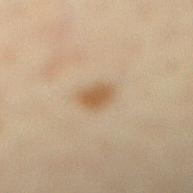<case>
  <biopsy_status>not biopsied; imaged during a skin examination</biopsy_status>
  <automated_metrics>
    <border_irregularity_0_10>2.0</border_irregularity_0_10>
    <color_variation_0_10>2.0</color_variation_0_10>
    <peripheral_color_asymmetry>0.5</peripheral_color_asymmetry>
  </automated_metrics>
  <patient>
    <sex>female</sex>
    <age_approx>65</age_approx>
  </patient>
  <site>right lower leg</site>
  <lesion_size>
    <long_diameter_mm_approx>2.5</long_diameter_mm_approx>
  </lesion_size>
  <image>
    <source>total-body photography crop</source>
    <field_of_view_mm>15</field_of_view_mm>
  </image>
  <lighting>cross-polarized</lighting>
</case>